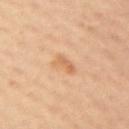Impression: The lesion was photographed on a routine skin check and not biopsied; there is no pathology result. Context: The subject is a female in their 40s. From the left arm. This is a cross-polarized tile. Approximately 3 mm at its widest. Automated tile analysis of the lesion measured an area of roughly 4 mm², an outline eccentricity of about 0.75 (0 = round, 1 = elongated), and a symmetry-axis asymmetry near 0.4. A region of skin cropped from a whole-body photographic capture, roughly 15 mm wide.